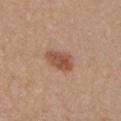  biopsy_status: not biopsied; imaged during a skin examination
  image:
    source: total-body photography crop
    field_of_view_mm: 15
  automated_metrics:
    border_irregularity_0_10: 2.0
    color_variation_0_10: 2.5
    peripheral_color_asymmetry: 1.0
    nevus_likeness_0_100: 95
    lesion_detection_confidence_0_100: 100
  lesion_size:
    long_diameter_mm_approx: 4.0
  patient:
    sex: male
    age_approx: 55
  site: arm